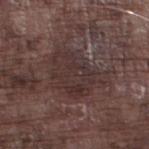follow-up = imaged on a skin check; not biopsied
patient = male, approximately 75 years of age
image = 15 mm crop, total-body photography
site = the left thigh
size = ~7 mm (longest diameter)
automated metrics = an average lesion color of about L≈32 a*≈16 b*≈16 (CIELAB), a lesion–skin lightness drop of about 6, and a normalized border contrast of about 6; border irregularity of about 4.5 on a 0–10 scale and radial color variation of about 1